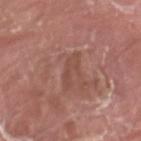The lesion is on the leg.
A male patient aged 38–42.
The lesion's longest dimension is about 4 mm.
A 15 mm close-up tile from a total-body photography series done for melanoma screening.